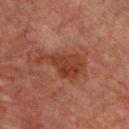Recorded during total-body skin imaging; not selected for excision or biopsy.
A close-up tile cropped from a whole-body skin photograph, about 15 mm across.
The lesion is located on the chest.
A male subject roughly 60 years of age.